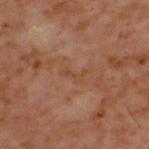Q: Was this lesion biopsied?
A: imaged on a skin check; not biopsied
Q: What is the anatomic site?
A: the upper back
Q: Who is the patient?
A: male, about 60 years old
Q: How large is the lesion?
A: ≈2.5 mm
Q: What is the imaging modality?
A: total-body-photography crop, ~15 mm field of view
Q: Illumination type?
A: cross-polarized illumination